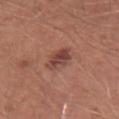Clinical impression: The lesion was tiled from a total-body skin photograph and was not biopsied. Acquisition and patient details: A lesion tile, about 15 mm wide, cut from a 3D total-body photograph. A male patient, in their mid- to late 20s. On the arm. Captured under white-light illumination. The total-body-photography lesion software estimated a footprint of about 6 mm², an outline eccentricity of about 0.4 (0 = round, 1 = elongated), and a symmetry-axis asymmetry near 0.25. And it measured border irregularity of about 2 on a 0–10 scale and a color-variation rating of about 4.5/10. Approximately 3 mm at its widest.